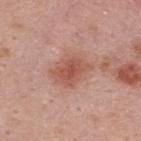Recorded during total-body skin imaging; not selected for excision or biopsy. Cropped from a total-body skin-imaging series; the visible field is about 15 mm. Automated tile analysis of the lesion measured a border-irregularity index near 3/10, a within-lesion color-variation index near 3/10, and radial color variation of about 1. A male patient, approximately 45 years of age. About 4 mm across. On the upper back. This is a white-light tile.